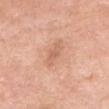{"biopsy_status": "not biopsied; imaged during a skin examination", "lesion_size": {"long_diameter_mm_approx": 3.5}, "image": {"source": "total-body photography crop", "field_of_view_mm": 15}, "site": "head or neck", "patient": {"sex": "female", "age_approx": 70}}